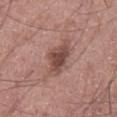| field | value |
|---|---|
| subject | male, in their 60s |
| image source | 15 mm crop, total-body photography |
| anatomic site | the abdomen |
| automated lesion analysis | roughly 11 lightness units darker than nearby skin |